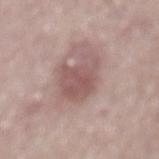Assessment: Captured during whole-body skin photography for melanoma surveillance; the lesion was not biopsied. Acquisition and patient details: This is a white-light tile. The lesion is on the mid back. A roughly 15 mm field-of-view crop from a total-body skin photograph. A male patient aged around 70. Automated tile analysis of the lesion measured roughly 10 lightness units darker than nearby skin and a normalized border contrast of about 7. And it measured a border-irregularity index near 3.5/10, internal color variation of about 3.5 on a 0–10 scale, and peripheral color asymmetry of about 1. And it measured a classifier nevus-likeness of about 25/100 and a detector confidence of about 100 out of 100 that the crop contains a lesion.